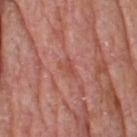The lesion was tiled from a total-body skin photograph and was not biopsied.
A male patient about 75 years old.
A region of skin cropped from a whole-body photographic capture, roughly 15 mm wide.
Approximately 2.5 mm at its widest.
From the head or neck.